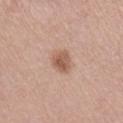Captured during whole-body skin photography for melanoma surveillance; the lesion was not biopsied. The lesion is on the right lower leg. The subject is a female approximately 55 years of age. Longest diameter approximately 3.5 mm. This is a white-light tile. A close-up tile cropped from a whole-body skin photograph, about 15 mm across. Automated tile analysis of the lesion measured an area of roughly 6.5 mm² and a shape-asymmetry score of about 0.15 (0 = symmetric).